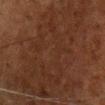biopsy status: catalogued during a skin exam; not biopsied
image source: total-body-photography crop, ~15 mm field of view
automated lesion analysis: a border-irregularity index near 8/10 and peripheral color asymmetry of about 1; a classifier nevus-likeness of about 0/100 and a detector confidence of about 65 out of 100 that the crop contains a lesion
patient: male, approximately 50 years of age
lesion diameter: ~15.5 mm (longest diameter)
anatomic site: the chest